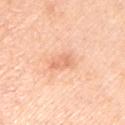* workup — imaged on a skin check; not biopsied
* lighting — white-light illumination
* image — ~15 mm crop, total-body skin-cancer survey
* lesion diameter — about 3 mm
* patient — male, aged approximately 50
* location — the left upper arm